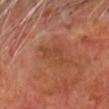Recorded during total-body skin imaging; not selected for excision or biopsy.
A close-up tile cropped from a whole-body skin photograph, about 15 mm across.
On the head or neck.
A male subject roughly 70 years of age.